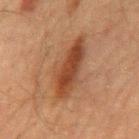Part of a total-body skin-imaging series; this lesion was reviewed on a skin check and was not flagged for biopsy. Cropped from a total-body skin-imaging series; the visible field is about 15 mm. Captured under cross-polarized illumination. The total-body-photography lesion software estimated a lesion area of about 17 mm² and a shape-asymmetry score of about 0.3 (0 = symmetric). The software also gave internal color variation of about 5 on a 0–10 scale and a peripheral color-asymmetry measure near 1.5. The analysis additionally found a classifier nevus-likeness of about 70/100 and a detector confidence of about 100 out of 100 that the crop contains a lesion. Located on the mid back. The subject is a male about 60 years old. Longest diameter approximately 7.5 mm.